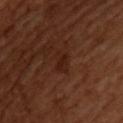Clinical impression:
Part of a total-body skin-imaging series; this lesion was reviewed on a skin check and was not flagged for biopsy.
Clinical summary:
The lesion is located on the upper back. Imaged with cross-polarized lighting. A male subject, in their 50s. This image is a 15 mm lesion crop taken from a total-body photograph. The lesion-visualizer software estimated an area of roughly 3.5 mm², an outline eccentricity of about 0.75 (0 = round, 1 = elongated), and a shape-asymmetry score of about 0.3 (0 = symmetric). It also reported a lesion color around L≈21 a*≈21 b*≈24 in CIELAB, a lesion–skin lightness drop of about 5, and a lesion-to-skin contrast of about 6.5 (normalized; higher = more distinct). And it measured a border-irregularity rating of about 3.5/10 and a color-variation rating of about 0.5/10. The lesion's longest dimension is about 2.5 mm.